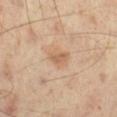Clinical impression: This lesion was catalogued during total-body skin photography and was not selected for biopsy. Image and clinical context: A 15 mm crop from a total-body photograph taken for skin-cancer surveillance. The lesion is on the leg. The subject is a male aged around 55.